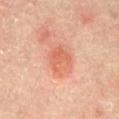| feature | finding |
|---|---|
| biopsy status | catalogued during a skin exam; not biopsied |
| imaging modality | ~15 mm tile from a whole-body skin photo |
| lighting | cross-polarized illumination |
| automated lesion analysis | an area of roughly 7.5 mm² and an outline eccentricity of about 0.5 (0 = round, 1 = elongated); a mean CIELAB color near L≈62 a*≈30 b*≈34 and roughly 8 lightness units darker than nearby skin |
| site | the abdomen |
| lesion size | about 3.5 mm |
| patient | male, aged 63 to 67 |